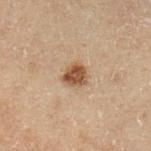The lesion was photographed on a routine skin check and not biopsied; there is no pathology result.
Captured under cross-polarized illumination.
Located on the left lower leg.
The lesion-visualizer software estimated a footprint of about 5.5 mm² and a shape eccentricity near 0.45. The software also gave a lesion color around L≈38 a*≈15 b*≈26 in CIELAB and a lesion–skin lightness drop of about 11.
A region of skin cropped from a whole-body photographic capture, roughly 15 mm wide.
The subject is a male aged 68 to 72.